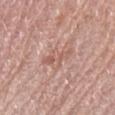This lesion was catalogued during total-body skin photography and was not selected for biopsy. A female subject approximately 65 years of age. Imaged with white-light lighting. An algorithmic analysis of the crop reported a lesion area of about 4.5 mm², a shape eccentricity near 0.9, and a shape-asymmetry score of about 0.4 (0 = symmetric). And it measured border irregularity of about 6 on a 0–10 scale, a color-variation rating of about 2.5/10, and peripheral color asymmetry of about 0.5. The lesion is on the left upper arm. The recorded lesion diameter is about 3.5 mm. A roughly 15 mm field-of-view crop from a total-body skin photograph.